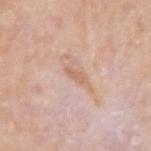A male patient, aged around 75.
Longest diameter approximately 2.5 mm.
The lesion-visualizer software estimated border irregularity of about 3.5 on a 0–10 scale, a within-lesion color-variation index near 0/10, and a peripheral color-asymmetry measure near 0. It also reported an automated nevus-likeness rating near 0 out of 100.
The lesion is located on the arm.
This is a white-light tile.
Cropped from a whole-body photographic skin survey; the tile spans about 15 mm.